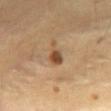Clinical impression:
Imaged during a routine full-body skin examination; the lesion was not biopsied and no histopathology is available.
Acquisition and patient details:
A close-up tile cropped from a whole-body skin photograph, about 15 mm across. A female patient, aged 38–42. From the abdomen. This is a cross-polarized tile.